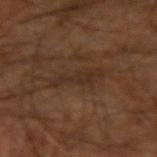No biopsy was performed on this lesion — it was imaged during a full skin examination and was not determined to be concerning. Imaged with cross-polarized lighting. About 5 mm across. This image is a 15 mm lesion crop taken from a total-body photograph. A male patient in their 60s. Located on the left arm.